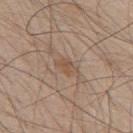{"biopsy_status": "not biopsied; imaged during a skin examination", "patient": {"sex": "male", "age_approx": 50}, "site": "mid back", "lighting": "white-light", "image": {"source": "total-body photography crop", "field_of_view_mm": 15}}